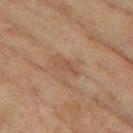Clinical impression:
The lesion was tiled from a total-body skin photograph and was not biopsied.
Image and clinical context:
On the left thigh. The tile uses cross-polarized illumination. A female subject, roughly 60 years of age. Longest diameter approximately 3 mm. Cropped from a whole-body photographic skin survey; the tile spans about 15 mm.